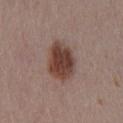Clinical impression:
Imaged during a routine full-body skin examination; the lesion was not biopsied and no histopathology is available.
Acquisition and patient details:
The subject is a male in their mid-50s. Automated tile analysis of the lesion measured border irregularity of about 1.5 on a 0–10 scale, a within-lesion color-variation index near 4.5/10, and a peripheral color-asymmetry measure near 1.5. The lesion is located on the chest. A close-up tile cropped from a whole-body skin photograph, about 15 mm across. This is a white-light tile. The recorded lesion diameter is about 5 mm.